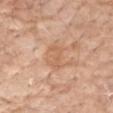{"biopsy_status": "not biopsied; imaged during a skin examination", "site": "arm", "patient": {"sex": "female", "age_approx": 65}, "image": {"source": "total-body photography crop", "field_of_view_mm": 15}, "automated_metrics": {"area_mm2_approx": 4.0, "shape_asymmetry": 0.4, "cielab_L": 61, "cielab_a": 22, "cielab_b": 34, "vs_skin_darker_L": 7.0, "vs_skin_contrast_norm": 5.0, "lesion_detection_confidence_0_100": 100}, "lesion_size": {"long_diameter_mm_approx": 3.0}}